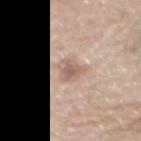| feature | finding |
|---|---|
| imaging modality | 15 mm crop, total-body photography |
| subject | male, approximately 50 years of age |
| diameter | ~3.5 mm (longest diameter) |
| lighting | white-light illumination |
| location | the mid back |
| automated metrics | roughly 10 lightness units darker than nearby skin and a normalized border contrast of about 6.5; border irregularity of about 3 on a 0–10 scale and radial color variation of about 1; an automated nevus-likeness rating near 30 out of 100 |